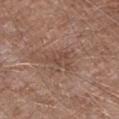workup = total-body-photography surveillance lesion; no biopsy | illumination = white-light | body site = the right lower leg | patient = male, in their 60s | imaging modality = ~15 mm crop, total-body skin-cancer survey | lesion diameter = about 3 mm.